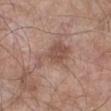  biopsy_status: not biopsied; imaged during a skin examination
  image:
    source: total-body photography crop
    field_of_view_mm: 15
  site: left lower leg
  patient:
    sex: male
    age_approx: 55